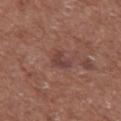Clinical impression:
Captured during whole-body skin photography for melanoma surveillance; the lesion was not biopsied.
Acquisition and patient details:
A 15 mm close-up tile from a total-body photography series done for melanoma screening. The subject is a male about 75 years old. The recorded lesion diameter is about 3 mm. On the front of the torso.